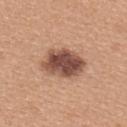Recorded during total-body skin imaging; not selected for excision or biopsy. A female subject approximately 30 years of age. From the upper back. The recorded lesion diameter is about 5.5 mm. The total-body-photography lesion software estimated an area of roughly 15 mm² and an outline eccentricity of about 0.75 (0 = round, 1 = elongated). The analysis additionally found an average lesion color of about L≈50 a*≈21 b*≈27 (CIELAB), roughly 17 lightness units darker than nearby skin, and a normalized lesion–skin contrast near 11.5. The analysis additionally found a classifier nevus-likeness of about 60/100 and lesion-presence confidence of about 100/100. A region of skin cropped from a whole-body photographic capture, roughly 15 mm wide.